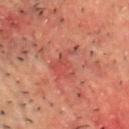patient: male, approximately 50 years of age
image: ~15 mm crop, total-body skin-cancer survey
TBP lesion metrics: a footprint of about 8 mm² and an eccentricity of roughly 0.75; an average lesion color of about L≈38 a*≈26 b*≈24 (CIELAB), a lesion–skin lightness drop of about 5, and a normalized border contrast of about 5; an automated nevus-likeness rating near 0 out of 100 and lesion-presence confidence of about 95/100
anatomic site: the front of the torso